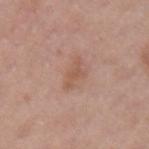Findings:
– biopsy status · total-body-photography surveillance lesion; no biopsy
– location · the left upper arm
– lighting · white-light illumination
– diameter · ≈3.5 mm
– image source · ~15 mm tile from a whole-body skin photo
– patient · female, about 40 years old